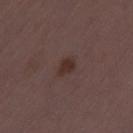Q: Was this lesion biopsied?
A: imaged on a skin check; not biopsied
Q: Who is the patient?
A: female, approximately 30 years of age
Q: How was the tile lit?
A: white-light illumination
Q: Lesion location?
A: the left thigh
Q: How was this image acquired?
A: ~15 mm crop, total-body skin-cancer survey
Q: What did automated image analysis measure?
A: an average lesion color of about L≈30 a*≈17 b*≈19 (CIELAB), a lesion–skin lightness drop of about 7, and a normalized lesion–skin contrast near 8; a border-irregularity rating of about 2.5/10, a color-variation rating of about 1/10, and radial color variation of about 0.5; a classifier nevus-likeness of about 90/100 and a lesion-detection confidence of about 100/100